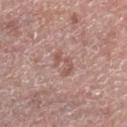No biopsy was performed on this lesion — it was imaged during a full skin examination and was not determined to be concerning.
A 15 mm close-up tile from a total-body photography series done for melanoma screening.
This is a white-light tile.
On the right lower leg.
The subject is a male aged around 75.